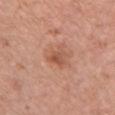Assessment: No biopsy was performed on this lesion — it was imaged during a full skin examination and was not determined to be concerning. Context: About 2.5 mm across. This is a white-light tile. A female subject, about 40 years old. A close-up tile cropped from a whole-body skin photograph, about 15 mm across. Located on the chest. Automated tile analysis of the lesion measured a classifier nevus-likeness of about 5/100 and a lesion-detection confidence of about 100/100.